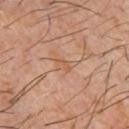Impression:
This lesion was catalogued during total-body skin photography and was not selected for biopsy.
Acquisition and patient details:
The lesion-visualizer software estimated a classifier nevus-likeness of about 0/100 and lesion-presence confidence of about 70/100. Cropped from a whole-body photographic skin survey; the tile spans about 15 mm. A male subject about 60 years old. The lesion is located on the front of the torso. Imaged with cross-polarized lighting.